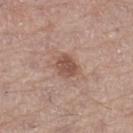| key | value |
|---|---|
| follow-up | total-body-photography surveillance lesion; no biopsy |
| size | ≈3 mm |
| acquisition | total-body-photography crop, ~15 mm field of view |
| subject | male, aged around 75 |
| body site | the left thigh |